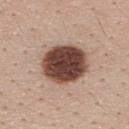This lesion was catalogued during total-body skin photography and was not selected for biopsy.
The tile uses white-light illumination.
An algorithmic analysis of the crop reported a footprint of about 22 mm², an eccentricity of roughly 0.45, and two-axis asymmetry of about 0.1. The software also gave a border-irregularity rating of about 1/10, internal color variation of about 5.5 on a 0–10 scale, and a peripheral color-asymmetry measure near 1.5.
The patient is a female in their mid- to late 20s.
A 15 mm crop from a total-body photograph taken for skin-cancer surveillance.
The lesion's longest dimension is about 5.5 mm.
On the upper back.